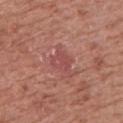No biopsy was performed on this lesion — it was imaged during a full skin examination and was not determined to be concerning. From the left upper arm. A male subject, in their mid-70s. A roughly 15 mm field-of-view crop from a total-body skin photograph.